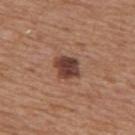Notes:
• biopsy status · imaged on a skin check; not biopsied
• anatomic site · the mid back
• diameter · ≈3.5 mm
• lighting · white-light
• subject · male, roughly 65 years of age
• acquisition · ~15 mm crop, total-body skin-cancer survey